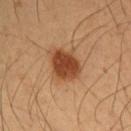This lesion was catalogued during total-body skin photography and was not selected for biopsy. Automated image analysis of the tile measured an automated nevus-likeness rating near 100 out of 100. Captured under cross-polarized illumination. On the right upper arm. A 15 mm close-up tile from a total-body photography series done for melanoma screening. A male patient aged around 55. The lesion's longest dimension is about 4 mm.